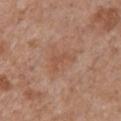notes = total-body-photography surveillance lesion; no biopsy | image = 15 mm crop, total-body photography | body site = the chest | automated lesion analysis = a footprint of about 3.5 mm², an eccentricity of roughly 0.85, and two-axis asymmetry of about 0.55; a lesion color around L≈52 a*≈22 b*≈31 in CIELAB and a normalized lesion–skin contrast near 5; a peripheral color-asymmetry measure near 0; a classifier nevus-likeness of about 0/100 and a detector confidence of about 100 out of 100 that the crop contains a lesion | subject = female, aged approximately 70 | lesion diameter = ≈3 mm.